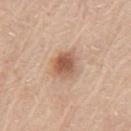notes=no biopsy performed (imaged during a skin exam)
patient=male, aged 78–82
lesion size=~3 mm (longest diameter)
image source=~15 mm crop, total-body skin-cancer survey
body site=the left upper arm
lighting=white-light illumination